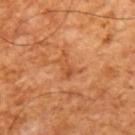Case summary:
- follow-up · imaged on a skin check; not biopsied
- acquisition · total-body-photography crop, ~15 mm field of view
- image-analysis metrics · a lesion area of about 4.5 mm², an eccentricity of roughly 0.85, and two-axis asymmetry of about 0.4
- subject · male, in their mid-60s
- diameter · about 3 mm